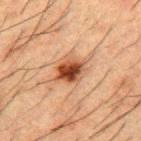Impression: Imaged during a routine full-body skin examination; the lesion was not biopsied and no histopathology is available. Context: Located on the mid back. A male patient, about 50 years old. An algorithmic analysis of the crop reported an area of roughly 9 mm² and a shape eccentricity near 0.55. It also reported roughly 14 lightness units darker than nearby skin and a normalized border contrast of about 11. A region of skin cropped from a whole-body photographic capture, roughly 15 mm wide. This is a cross-polarized tile. Approximately 3.5 mm at its widest.